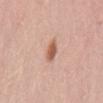Q: Illumination type?
A: white-light
Q: Patient demographics?
A: female, approximately 50 years of age
Q: Where on the body is the lesion?
A: the mid back
Q: What is the imaging modality?
A: 15 mm crop, total-body photography
Q: What did automated image analysis measure?
A: a border-irregularity index near 2/10, a color-variation rating of about 3/10, and peripheral color asymmetry of about 1; a classifier nevus-likeness of about 95/100 and a detector confidence of about 100 out of 100 that the crop contains a lesion
Q: How large is the lesion?
A: ≈2.5 mm